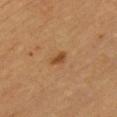<lesion>
<biopsy_status>not biopsied; imaged during a skin examination</biopsy_status>
<image>
  <source>total-body photography crop</source>
  <field_of_view_mm>15</field_of_view_mm>
</image>
<site>chest</site>
<lighting>cross-polarized</lighting>
<lesion_size>
  <long_diameter_mm_approx>2.5</long_diameter_mm_approx>
</lesion_size>
<patient>
  <sex>female</sex>
  <age_approx>45</age_approx>
</patient>
</lesion>